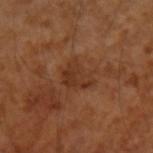{"biopsy_status": "not biopsied; imaged during a skin examination", "site": "left forearm", "patient": {"sex": "male", "age_approx": 55}, "image": {"source": "total-body photography crop", "field_of_view_mm": 15}, "lighting": "cross-polarized", "lesion_size": {"long_diameter_mm_approx": 3.5}}